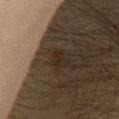The lesion was tiled from a total-body skin photograph and was not biopsied.
Captured under cross-polarized illumination.
Approximately 2.5 mm at its widest.
Located on the front of the torso.
A male patient, aged 43–47.
Automated image analysis of the tile measured a lesion area of about 3 mm², a shape eccentricity near 0.85, and a symmetry-axis asymmetry near 0.4. The software also gave roughly 4 lightness units darker than nearby skin and a normalized lesion–skin contrast near 6. It also reported border irregularity of about 4 on a 0–10 scale and a within-lesion color-variation index near 2/10. It also reported a classifier nevus-likeness of about 0/100 and lesion-presence confidence of about 100/100.
A close-up tile cropped from a whole-body skin photograph, about 15 mm across.